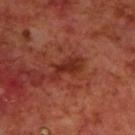Notes:
– location — the upper back
– subject — male, in their 70s
– image — 15 mm crop, total-body photography
– lesion diameter — about 4 mm